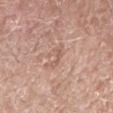Q: Is there a histopathology result?
A: total-body-photography surveillance lesion; no biopsy
Q: Where on the body is the lesion?
A: the right forearm
Q: What is the imaging modality?
A: total-body-photography crop, ~15 mm field of view
Q: Who is the patient?
A: female, about 55 years old